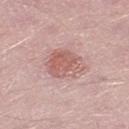Background:
A male subject about 50 years old. Cropped from a total-body skin-imaging series; the visible field is about 15 mm. The lesion is on the right thigh.